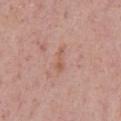biopsy status: imaged on a skin check; not biopsied | location: the chest | acquisition: ~15 mm crop, total-body skin-cancer survey | patient: male, in their mid-70s.